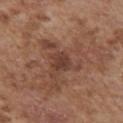Case summary:
* biopsy status — total-body-photography surveillance lesion; no biopsy
* lighting — white-light illumination
* body site — the chest
* subject — male, in their mid- to late 70s
* imaging modality — total-body-photography crop, ~15 mm field of view
* diameter — ≈2.5 mm
* automated metrics — an area of roughly 4.5 mm², an eccentricity of roughly 0.55, and a shape-asymmetry score of about 0.25 (0 = symmetric); a border-irregularity index near 2.5/10 and peripheral color asymmetry of about 1; lesion-presence confidence of about 100/100